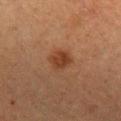Q: Was this lesion biopsied?
A: imaged on a skin check; not biopsied
Q: What did automated image analysis measure?
A: an area of roughly 5.5 mm² and a shape eccentricity near 0.65; a border-irregularity index near 2.5/10, a within-lesion color-variation index near 2.5/10, and a peripheral color-asymmetry measure near 1; a classifier nevus-likeness of about 85/100 and a detector confidence of about 100 out of 100 that the crop contains a lesion
Q: What is the lesion's diameter?
A: ≈3 mm
Q: Patient demographics?
A: female, aged 58–62
Q: How was the tile lit?
A: cross-polarized illumination
Q: Lesion location?
A: the left forearm
Q: What is the imaging modality?
A: 15 mm crop, total-body photography